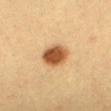* workup · total-body-photography surveillance lesion; no biopsy
* automated metrics · an area of roughly 9 mm², a shape eccentricity near 0.65, and two-axis asymmetry of about 0.15; an average lesion color of about L≈50 a*≈21 b*≈37 (CIELAB), roughly 17 lightness units darker than nearby skin, and a normalized lesion–skin contrast near 11.5
* subject · female, aged around 30
* illumination · cross-polarized illumination
* imaging modality · total-body-photography crop, ~15 mm field of view
* diameter · ~4 mm (longest diameter)
* anatomic site · the mid back